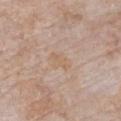The lesion was photographed on a routine skin check and not biopsied; there is no pathology result.